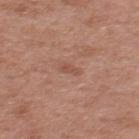Recorded during total-body skin imaging; not selected for excision or biopsy.